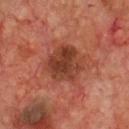Notes:
– notes · catalogued during a skin exam; not biopsied
– patient · male, aged 68 to 72
– lighting · cross-polarized illumination
– location · the chest
– imaging modality · ~15 mm crop, total-body skin-cancer survey